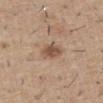– follow-up · imaged on a skin check; not biopsied
– location · the abdomen
– TBP lesion metrics · a footprint of about 5.5 mm², a shape eccentricity near 0.65, and two-axis asymmetry of about 0.15; a lesion color around L≈52 a*≈17 b*≈29 in CIELAB, a lesion–skin lightness drop of about 11, and a lesion-to-skin contrast of about 8 (normalized; higher = more distinct); border irregularity of about 2 on a 0–10 scale, internal color variation of about 2.5 on a 0–10 scale, and peripheral color asymmetry of about 1
– imaging modality · ~15 mm crop, total-body skin-cancer survey
– patient · male, aged around 60
– size · ≈3 mm
– illumination · white-light